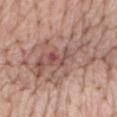Captured during whole-body skin photography for melanoma surveillance; the lesion was not biopsied.
Captured under white-light illumination.
A female subject, in their mid-70s.
The lesion is located on the mid back.
Cropped from a whole-body photographic skin survey; the tile spans about 15 mm.
Longest diameter approximately 10.5 mm.